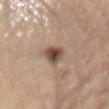The patient is a female aged approximately 65.
A close-up tile cropped from a whole-body skin photograph, about 15 mm across.
The lesion is on the abdomen.
About 4 mm across.
Imaged with white-light lighting.